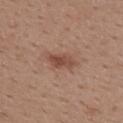follow-up: imaged on a skin check; not biopsied | subject: female, in their mid-20s | anatomic site: the back | size: ≈3.5 mm | lighting: white-light | image source: total-body-photography crop, ~15 mm field of view | automated metrics: a lesion-detection confidence of about 100/100.